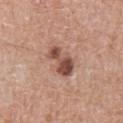biopsy_status: not biopsied; imaged during a skin examination
patient:
  sex: male
  age_approx: 75
image:
  source: total-body photography crop
  field_of_view_mm: 15
site: front of the torso